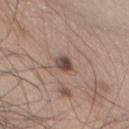Q: Was this lesion biopsied?
A: total-body-photography surveillance lesion; no biopsy
Q: How was the tile lit?
A: white-light illumination
Q: What is the anatomic site?
A: the chest
Q: Patient demographics?
A: male, approximately 65 years of age
Q: What did automated image analysis measure?
A: a mean CIELAB color near L≈46 a*≈17 b*≈23, about 13 CIELAB-L* units darker than the surrounding skin, and a lesion-to-skin contrast of about 9.5 (normalized; higher = more distinct)
Q: What kind of image is this?
A: total-body-photography crop, ~15 mm field of view